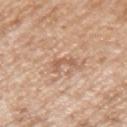{"biopsy_status": "not biopsied; imaged during a skin examination", "lesion_size": {"long_diameter_mm_approx": 2.5}, "image": {"source": "total-body photography crop", "field_of_view_mm": 15}, "automated_metrics": {"cielab_L": 59, "cielab_a": 21, "cielab_b": 32, "vs_skin_darker_L": 9.0, "vs_skin_contrast_norm": 6.0, "border_irregularity_0_10": 8.0, "color_variation_0_10": 0.0, "peripheral_color_asymmetry": 0.0, "nevus_likeness_0_100": 0, "lesion_detection_confidence_0_100": 100}, "patient": {"sex": "male", "age_approx": 65}, "lighting": "white-light", "site": "left upper arm"}